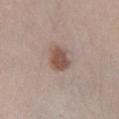{
  "biopsy_status": "not biopsied; imaged during a skin examination",
  "patient": {
    "sex": "female",
    "age_approx": 40
  },
  "site": "left lower leg",
  "automated_metrics": {
    "cielab_L": 51,
    "cielab_a": 19,
    "cielab_b": 24,
    "vs_skin_darker_L": 12.0,
    "vs_skin_contrast_norm": 9.0,
    "border_irregularity_0_10": 2.0,
    "color_variation_0_10": 2.0,
    "peripheral_color_asymmetry": 1.0,
    "lesion_detection_confidence_0_100": 100
  },
  "image": {
    "source": "total-body photography crop",
    "field_of_view_mm": 15
  },
  "lighting": "white-light"
}